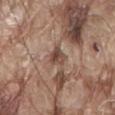• workup: imaged on a skin check; not biopsied
• subject: male, aged around 80
• imaging modality: 15 mm crop, total-body photography
• lighting: white-light illumination
• lesion diameter: about 2.5 mm
• site: the front of the torso